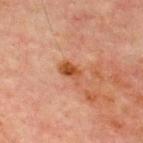• workup: catalogued during a skin exam; not biopsied